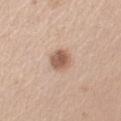Impression: Part of a total-body skin-imaging series; this lesion was reviewed on a skin check and was not flagged for biopsy. Image and clinical context: The lesion is on the left upper arm. A female patient, roughly 55 years of age. Cropped from a total-body skin-imaging series; the visible field is about 15 mm. Automated image analysis of the tile measured a lesion area of about 6 mm², a shape eccentricity near 0.45, and two-axis asymmetry of about 0.2. And it measured roughly 13 lightness units darker than nearby skin and a lesion-to-skin contrast of about 8.5 (normalized; higher = more distinct). The tile uses white-light illumination. The recorded lesion diameter is about 3 mm.